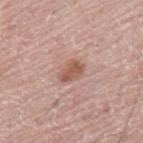Impression:
The lesion was photographed on a routine skin check and not biopsied; there is no pathology result.
Context:
Cropped from a whole-body photographic skin survey; the tile spans about 15 mm. An algorithmic analysis of the crop reported a footprint of about 5 mm², a shape eccentricity near 0.8, and a symmetry-axis asymmetry near 0.2. And it measured a lesion color around L≈55 a*≈22 b*≈27 in CIELAB, a lesion–skin lightness drop of about 11, and a normalized lesion–skin contrast near 7.5. And it measured a classifier nevus-likeness of about 80/100 and a detector confidence of about 100 out of 100 that the crop contains a lesion. From the mid back. Measured at roughly 3 mm in maximum diameter. A male patient, in their mid-70s.